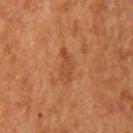biopsy_status: not biopsied; imaged during a skin examination
patient:
  sex: male
  age_approx: 50
site: upper back
image:
  source: total-body photography crop
  field_of_view_mm: 15